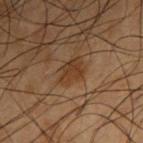Impression: Recorded during total-body skin imaging; not selected for excision or biopsy. Context: From the arm. Cropped from a whole-body photographic skin survey; the tile spans about 15 mm. The patient is a male roughly 50 years of age. This is a cross-polarized tile. Measured at roughly 2.5 mm in maximum diameter.